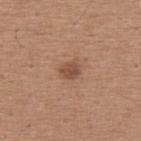{
  "biopsy_status": "not biopsied; imaged during a skin examination",
  "lesion_size": {
    "long_diameter_mm_approx": 2.5
  },
  "image": {
    "source": "total-body photography crop",
    "field_of_view_mm": 15
  },
  "site": "upper back",
  "patient": {
    "sex": "male",
    "age_approx": 65
  }
}